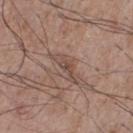Impression:
No biopsy was performed on this lesion — it was imaged during a full skin examination and was not determined to be concerning.
Context:
Located on the front of the torso. Cropped from a whole-body photographic skin survey; the tile spans about 15 mm. A male patient, in their mid- to late 70s.